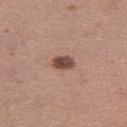Q: Lesion location?
A: the right thigh
Q: Lesion size?
A: ≈2.5 mm
Q: Patient demographics?
A: female, aged 33 to 37
Q: What is the imaging modality?
A: ~15 mm tile from a whole-body skin photo